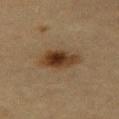notes: catalogued during a skin exam; not biopsied | site: the leg | tile lighting: cross-polarized illumination | image source: ~15 mm tile from a whole-body skin photo | size: ≈5 mm | subject: female, aged 53–57.